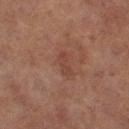Impression: The lesion was photographed on a routine skin check and not biopsied; there is no pathology result. Image and clinical context: Imaged with cross-polarized lighting. The total-body-photography lesion software estimated an area of roughly 3 mm², an eccentricity of roughly 0.9, and a symmetry-axis asymmetry near 0.45. The software also gave a lesion color around L≈36 a*≈21 b*≈24 in CIELAB and a lesion-to-skin contrast of about 5 (normalized; higher = more distinct). The software also gave a within-lesion color-variation index near 0/10 and peripheral color asymmetry of about 0. The subject is a female aged approximately 60. A close-up tile cropped from a whole-body skin photograph, about 15 mm across. The recorded lesion diameter is about 2.5 mm. The lesion is on the left thigh.